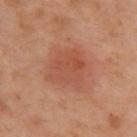No biopsy was performed on this lesion — it was imaged during a full skin examination and was not determined to be concerning. About 4.5 mm across. The total-body-photography lesion software estimated an area of roughly 14 mm², a shape eccentricity near 0.6, and a symmetry-axis asymmetry near 0.2. A 15 mm crop from a total-body photograph taken for skin-cancer surveillance. A female patient aged 53–57. The lesion is on the upper back.